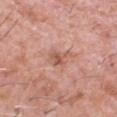The lesion was tiled from a total-body skin photograph and was not biopsied. Cropped from a whole-body photographic skin survey; the tile spans about 15 mm. Approximately 3.5 mm at its widest. The tile uses white-light illumination. The lesion is on the head or neck. A male patient, aged 58–62.